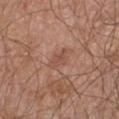Clinical impression: No biopsy was performed on this lesion — it was imaged during a full skin examination and was not determined to be concerning. Context: The patient is a male roughly 65 years of age. An algorithmic analysis of the crop reported an area of roughly 3.5 mm², an eccentricity of roughly 0.8, and two-axis asymmetry of about 0.35. It also reported a normalized lesion–skin contrast near 5.5. The lesion is on the chest. A 15 mm close-up tile from a total-body photography series done for melanoma screening. Measured at roughly 3 mm in maximum diameter. This is a white-light tile.